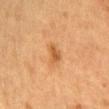Case summary:
- biopsy status — no biopsy performed (imaged during a skin exam)
- lesion diameter — about 3 mm
- image — ~15 mm crop, total-body skin-cancer survey
- anatomic site — the arm
- tile lighting — cross-polarized illumination
- patient — female, aged around 55